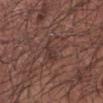anatomic site: the left forearm
acquisition: 15 mm crop, total-body photography
TBP lesion metrics: a shape-asymmetry score of about 0.4 (0 = symmetric); a lesion color around L≈36 a*≈18 b*≈21 in CIELAB, a lesion–skin lightness drop of about 6, and a normalized lesion–skin contrast near 5.5; border irregularity of about 5 on a 0–10 scale and internal color variation of about 3 on a 0–10 scale; a classifier nevus-likeness of about 0/100 and lesion-presence confidence of about 75/100
illumination: white-light illumination
subject: male, roughly 55 years of age